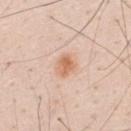Recorded during total-body skin imaging; not selected for excision or biopsy.
A male subject aged 33–37.
This image is a 15 mm lesion crop taken from a total-body photograph.
On the mid back.